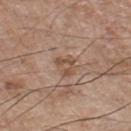biopsy status=catalogued during a skin exam; not biopsied | patient=male, aged 73 to 77 | image=total-body-photography crop, ~15 mm field of view | diameter=~2.5 mm (longest diameter) | site=the chest | lighting=white-light illumination.